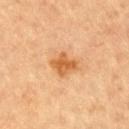Case summary:
- biopsy status · no biopsy performed (imaged during a skin exam)
- lighting · cross-polarized
- diameter · about 3.5 mm
- acquisition · ~15 mm crop, total-body skin-cancer survey
- location · the right upper arm
- subject · male, approximately 85 years of age or older